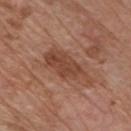follow-up — no biopsy performed (imaged during a skin exam); subject — male, aged 73–77; lesion diameter — about 6.5 mm; illumination — white-light illumination; acquisition — ~15 mm tile from a whole-body skin photo; location — the chest.